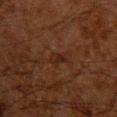Part of a total-body skin-imaging series; this lesion was reviewed on a skin check and was not flagged for biopsy. The patient is a male approximately 60 years of age. Measured at roughly 3 mm in maximum diameter. A lesion tile, about 15 mm wide, cut from a 3D total-body photograph. On the front of the torso.